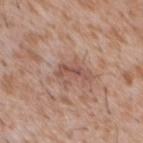biopsy status = total-body-photography surveillance lesion; no biopsy
subject = male, approximately 45 years of age
location = the chest
acquisition = 15 mm crop, total-body photography